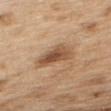- workup — imaged on a skin check; not biopsied
- subject — male, approximately 70 years of age
- image source — 15 mm crop, total-body photography
- anatomic site — the back